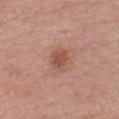This lesion was catalogued during total-body skin photography and was not selected for biopsy.
The lesion is located on the chest.
A male subject, aged around 60.
The lesion-visualizer software estimated a lesion color around L≈52 a*≈24 b*≈29 in CIELAB and a lesion–skin lightness drop of about 9. The analysis additionally found a classifier nevus-likeness of about 70/100 and a detector confidence of about 100 out of 100 that the crop contains a lesion.
Captured under white-light illumination.
Approximately 2.5 mm at its widest.
A roughly 15 mm field-of-view crop from a total-body skin photograph.